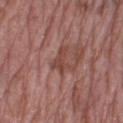{
  "biopsy_status": "not biopsied; imaged during a skin examination",
  "automated_metrics": {
    "border_irregularity_0_10": 7.5,
    "color_variation_0_10": 2.0,
    "peripheral_color_asymmetry": 1.0,
    "lesion_detection_confidence_0_100": 80
  },
  "patient": {
    "sex": "female",
    "age_approx": 70
  },
  "image": {
    "source": "total-body photography crop",
    "field_of_view_mm": 15
  },
  "site": "left thigh"
}